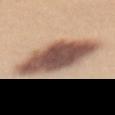| field | value |
|---|---|
| workup | no biopsy performed (imaged during a skin exam) |
| patient | female, aged approximately 25 |
| illumination | white-light illumination |
| acquisition | ~15 mm tile from a whole-body skin photo |
| location | the mid back |
| automated lesion analysis | a mean CIELAB color near L≈54 a*≈18 b*≈26, roughly 19 lightness units darker than nearby skin, and a lesion-to-skin contrast of about 12.5 (normalized; higher = more distinct); internal color variation of about 7.5 on a 0–10 scale and a peripheral color-asymmetry measure near 2 |